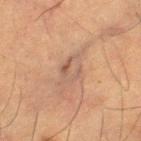Q: Is there a histopathology result?
A: total-body-photography surveillance lesion; no biopsy
Q: What is the imaging modality?
A: ~15 mm crop, total-body skin-cancer survey
Q: What is the anatomic site?
A: the right thigh
Q: How large is the lesion?
A: about 3 mm
Q: Who is the patient?
A: male, aged approximately 65